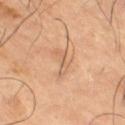notes: catalogued during a skin exam; not biopsied.